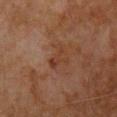Impression:
This lesion was catalogued during total-body skin photography and was not selected for biopsy.
Image and clinical context:
About 3 mm across. Located on the chest. Cropped from a whole-body photographic skin survey; the tile spans about 15 mm. A male subject, roughly 65 years of age. The lesion-visualizer software estimated a lesion color around L≈30 a*≈18 b*≈25 in CIELAB, about 5 CIELAB-L* units darker than the surrounding skin, and a normalized border contrast of about 5.5. It also reported an automated nevus-likeness rating near 0 out of 100 and a detector confidence of about 100 out of 100 that the crop contains a lesion.